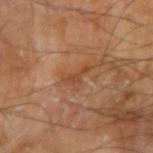biopsy_status: not biopsied; imaged during a skin examination
image:
  source: total-body photography crop
  field_of_view_mm: 15
patient:
  sex: male
  age_approx: 65
lesion_size:
  long_diameter_mm_approx: 3.5
site: right upper arm